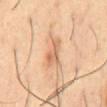The lesion's longest dimension is about 3.5 mm.
A roughly 15 mm field-of-view crop from a total-body skin photograph.
The subject is a male in their mid-50s.
On the abdomen.
The total-body-photography lesion software estimated a border-irregularity rating of about 4/10, a color-variation rating of about 2.5/10, and peripheral color asymmetry of about 1. And it measured a classifier nevus-likeness of about 20/100 and a detector confidence of about 100 out of 100 that the crop contains a lesion.
This is a cross-polarized tile.